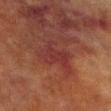Q: What is the imaging modality?
A: ~15 mm crop, total-body skin-cancer survey
Q: Patient demographics?
A: male, aged around 70
Q: How large is the lesion?
A: about 3.5 mm
Q: Illumination type?
A: cross-polarized illumination
Q: Where on the body is the lesion?
A: the right lower leg